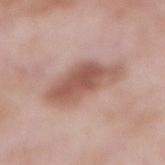notes: imaged on a skin check; not biopsied | acquisition: total-body-photography crop, ~15 mm field of view | anatomic site: the abdomen | patient: male, aged 53–57 | size: ~7 mm (longest diameter).